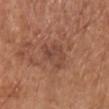The lesion was photographed on a routine skin check and not biopsied; there is no pathology result. From the chest. The patient is a female in their mid-70s. The tile uses white-light illumination. Longest diameter approximately 3.5 mm. A 15 mm close-up tile from a total-body photography series done for melanoma screening.